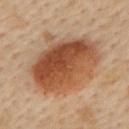Q: Was this lesion biopsied?
A: no biopsy performed (imaged during a skin exam)
Q: How was this image acquired?
A: ~15 mm tile from a whole-body skin photo
Q: What are the patient's age and sex?
A: female, about 45 years old
Q: How was the tile lit?
A: cross-polarized illumination
Q: What did automated image analysis measure?
A: a lesion area of about 41 mm² and an eccentricity of roughly 0.65; a lesion color around L≈52 a*≈24 b*≈36 in CIELAB, about 15 CIELAB-L* units darker than the surrounding skin, and a normalized border contrast of about 10.5; a border-irregularity rating of about 2.5/10, internal color variation of about 9 on a 0–10 scale, and a peripheral color-asymmetry measure near 3
Q: Lesion size?
A: ~8 mm (longest diameter)
Q: Lesion location?
A: the upper back